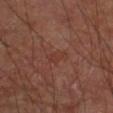Imaged during a routine full-body skin examination; the lesion was not biopsied and no histopathology is available. A male patient, approximately 60 years of age. A 15 mm crop from a total-body photograph taken for skin-cancer surveillance. This is a cross-polarized tile. About 3 mm across. On the arm. An algorithmic analysis of the crop reported a symmetry-axis asymmetry near 0.3. The analysis additionally found a mean CIELAB color near L≈32 a*≈20 b*≈24, a lesion–skin lightness drop of about 4, and a normalized border contrast of about 4.5. And it measured a border-irregularity index near 2.5/10 and a color-variation rating of about 2/10. And it measured a nevus-likeness score of about 0/100.